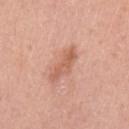Part of a total-body skin-imaging series; this lesion was reviewed on a skin check and was not flagged for biopsy.
This image is a 15 mm lesion crop taken from a total-body photograph.
A female patient, in their 40s.
Located on the arm.
The lesion's longest dimension is about 4.5 mm.
The total-body-photography lesion software estimated an outline eccentricity of about 0.9 (0 = round, 1 = elongated) and two-axis asymmetry of about 0.4. The analysis additionally found a color-variation rating of about 2.5/10 and a peripheral color-asymmetry measure near 1. The software also gave a classifier nevus-likeness of about 5/100 and a lesion-detection confidence of about 100/100.
This is a white-light tile.